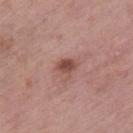Notes:
• follow-up · catalogued during a skin exam; not biopsied